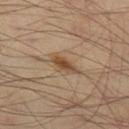Recorded during total-body skin imaging; not selected for excision or biopsy. Longest diameter approximately 4 mm. The subject is a male roughly 40 years of age. A region of skin cropped from a whole-body photographic capture, roughly 15 mm wide. The lesion is on the left thigh. Automated image analysis of the tile measured an area of roughly 5.5 mm² and a symmetry-axis asymmetry near 0.3. The analysis additionally found border irregularity of about 3.5 on a 0–10 scale, a color-variation rating of about 4/10, and a peripheral color-asymmetry measure near 1. The software also gave a nevus-likeness score of about 90/100 and lesion-presence confidence of about 100/100. Captured under cross-polarized illumination.